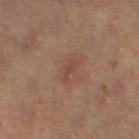Impression: Part of a total-body skin-imaging series; this lesion was reviewed on a skin check and was not flagged for biopsy. Background: The total-body-photography lesion software estimated an average lesion color of about L≈44 a*≈22 b*≈26 (CIELAB), a lesion–skin lightness drop of about 6, and a normalized lesion–skin contrast near 5. It also reported an automated nevus-likeness rating near 0 out of 100 and a detector confidence of about 100 out of 100 that the crop contains a lesion. Captured under cross-polarized illumination. This image is a 15 mm lesion crop taken from a total-body photograph. The lesion's longest dimension is about 2.5 mm. The lesion is on the left thigh. A female subject, aged 38 to 42.